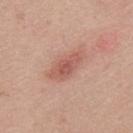biopsy status — imaged on a skin check; not biopsied
subject — male, aged 23 to 27
TBP lesion metrics — about 10 CIELAB-L* units darker than the surrounding skin and a lesion-to-skin contrast of about 6.5 (normalized; higher = more distinct); a border-irregularity rating of about 3/10, a color-variation rating of about 3.5/10, and radial color variation of about 1
lighting — white-light
lesion size — ~4.5 mm (longest diameter)
body site — the upper back
acquisition — total-body-photography crop, ~15 mm field of view